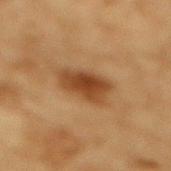Q: Is there a histopathology result?
A: catalogued during a skin exam; not biopsied
Q: Where on the body is the lesion?
A: the back
Q: What lighting was used for the tile?
A: cross-polarized illumination
Q: What is the lesion's diameter?
A: about 5 mm
Q: Who is the patient?
A: male, aged 83 or older
Q: What kind of image is this?
A: ~15 mm crop, total-body skin-cancer survey